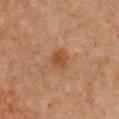About 2.5 mm across.
On the front of the torso.
A 15 mm crop from a total-body photograph taken for skin-cancer surveillance.
The patient is a female about 40 years old.